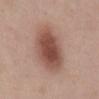Imaged during a routine full-body skin examination; the lesion was not biopsied and no histopathology is available.
A 15 mm close-up extracted from a 3D total-body photography capture.
The patient is a male aged 58–62.
Located on the abdomen.